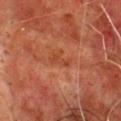{"biopsy_status": "not biopsied; imaged during a skin examination", "lighting": "cross-polarized", "patient": {"sex": "male", "age_approx": 65}, "lesion_size": {"long_diameter_mm_approx": 3.0}, "image": {"source": "total-body photography crop", "field_of_view_mm": 15}, "automated_metrics": {"cielab_L": 36, "cielab_a": 25, "cielab_b": 30, "vs_skin_darker_L": 5.0, "vs_skin_contrast_norm": 5.0, "border_irregularity_0_10": 4.5, "color_variation_0_10": 0.0, "peripheral_color_asymmetry": 0.0}, "site": "front of the torso"}